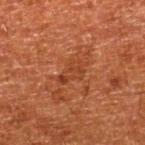Impression: The lesion was tiled from a total-body skin photograph and was not biopsied. Context: Automated image analysis of the tile measured a footprint of about 5.5 mm², a shape eccentricity near 0.85, and a symmetry-axis asymmetry near 0.35. It also reported an automated nevus-likeness rating near 0 out of 100 and a lesion-detection confidence of about 100/100. Approximately 3.5 mm at its widest. The patient is a male aged approximately 80. From the right lower leg. A 15 mm close-up extracted from a 3D total-body photography capture. Imaged with cross-polarized lighting.